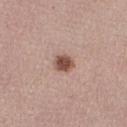Clinical impression:
Part of a total-body skin-imaging series; this lesion was reviewed on a skin check and was not flagged for biopsy.
Acquisition and patient details:
Imaged with white-light lighting. A female patient in their 50s. About 2.5 mm across. The lesion is on the right thigh. An algorithmic analysis of the crop reported an area of roughly 4.5 mm², a shape eccentricity near 0.65, and a shape-asymmetry score of about 0.2 (0 = symmetric). The analysis additionally found about 15 CIELAB-L* units darker than the surrounding skin and a normalized lesion–skin contrast near 10. The software also gave border irregularity of about 1.5 on a 0–10 scale and a color-variation rating of about 2.5/10. A 15 mm close-up extracted from a 3D total-body photography capture.